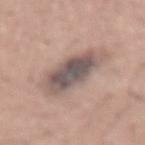| key | value |
|---|---|
| follow-up | catalogued during a skin exam; not biopsied |
| image source | ~15 mm crop, total-body skin-cancer survey |
| location | the lower back |
| size | ≈6 mm |
| patient | male, about 45 years old |